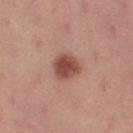Assessment:
The lesion was photographed on a routine skin check and not biopsied; there is no pathology result.
Clinical summary:
The subject is a female aged 23 to 27. A roughly 15 mm field-of-view crop from a total-body skin photograph. An algorithmic analysis of the crop reported a lesion color around L≈48 a*≈25 b*≈26 in CIELAB. The software also gave border irregularity of about 2 on a 0–10 scale, internal color variation of about 3.5 on a 0–10 scale, and a peripheral color-asymmetry measure near 1. The lesion is located on the left thigh.